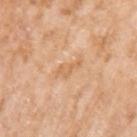Part of a total-body skin-imaging series; this lesion was reviewed on a skin check and was not flagged for biopsy.
Approximately 3.5 mm at its widest.
The lesion is located on the arm.
A female patient in their mid-70s.
A 15 mm crop from a total-body photograph taken for skin-cancer surveillance.
This is a white-light tile.
The total-body-photography lesion software estimated a within-lesion color-variation index near 1/10 and a peripheral color-asymmetry measure near 0.